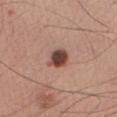Acquisition and patient details:
The total-body-photography lesion software estimated a color-variation rating of about 3.5/10 and peripheral color asymmetry of about 1. And it measured a classifier nevus-likeness of about 85/100 and a lesion-detection confidence of about 100/100. About 3 mm across. Cropped from a whole-body photographic skin survey; the tile spans about 15 mm. The lesion is on the arm. Imaged with white-light lighting. The subject is a male aged approximately 60.